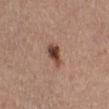| field | value |
|---|---|
| workup | no biopsy performed (imaged during a skin exam) |
| diameter | ~3 mm (longest diameter) |
| lighting | white-light illumination |
| subject | female, approximately 55 years of age |
| image source | 15 mm crop, total-body photography |
| body site | the leg |